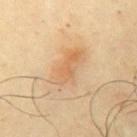biopsy status=catalogued during a skin exam; not biopsied | size=about 5 mm | site=the left upper arm | automated lesion analysis=a mean CIELAB color near L≈62 a*≈18 b*≈36, a lesion–skin lightness drop of about 7, and a normalized border contrast of about 5; a border-irregularity rating of about 2.5/10, a within-lesion color-variation index near 5/10, and peripheral color asymmetry of about 1.5 | subject=male, aged approximately 65 | illumination=cross-polarized illumination | acquisition=15 mm crop, total-body photography.